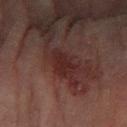Clinical impression: No biopsy was performed on this lesion — it was imaged during a full skin examination and was not determined to be concerning. Clinical summary: A female subject, in their 80s. A roughly 15 mm field-of-view crop from a total-body skin photograph. On the left forearm. Captured under cross-polarized illumination. About 4 mm across.